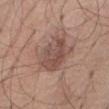Image and clinical context:
Approximately 5 mm at its widest. A male patient aged around 70. A lesion tile, about 15 mm wide, cut from a 3D total-body photograph. The lesion is located on the right thigh. Captured under white-light illumination.